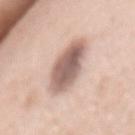{"biopsy_status": "not biopsied; imaged during a skin examination", "patient": {"sex": "female", "age_approx": 50}, "site": "mid back", "image": {"source": "total-body photography crop", "field_of_view_mm": 15}}